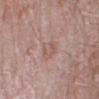The lesion is on the left lower leg. About 3 mm across. The patient is a male aged around 85 or older. A roughly 15 mm field-of-view crop from a total-body skin photograph. Automated tile analysis of the lesion measured a lesion area of about 4.5 mm², an eccentricity of roughly 0.7, and a symmetry-axis asymmetry near 0.25. The software also gave a mean CIELAB color near L≈56 a*≈19 b*≈24, roughly 6 lightness units darker than nearby skin, and a lesion-to-skin contrast of about 5 (normalized; higher = more distinct). The software also gave border irregularity of about 2.5 on a 0–10 scale, a within-lesion color-variation index near 2.5/10, and peripheral color asymmetry of about 1. The analysis additionally found a nevus-likeness score of about 0/100 and a detector confidence of about 100 out of 100 that the crop contains a lesion. Captured under white-light illumination.